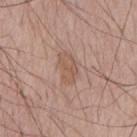Case summary:
• biopsy status — catalogued during a skin exam; not biopsied